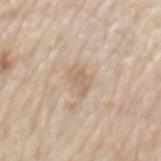| feature | finding |
|---|---|
| biopsy status | no biopsy performed (imaged during a skin exam) |
| site | the mid back |
| illumination | white-light |
| subject | male, approximately 80 years of age |
| lesion size | ≈3.5 mm |
| image source | ~15 mm crop, total-body skin-cancer survey |